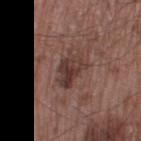Captured under white-light illumination. A male subject, in their 50s. Located on the right thigh. The lesion's longest dimension is about 4 mm. A lesion tile, about 15 mm wide, cut from a 3D total-body photograph.